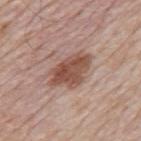{
  "biopsy_status": "not biopsied; imaged during a skin examination",
  "site": "mid back",
  "automated_metrics": {
    "area_mm2_approx": 12.0,
    "shape_asymmetry": 0.25,
    "vs_skin_darker_L": 12.0,
    "vs_skin_contrast_norm": 9.0,
    "border_irregularity_0_10": 3.0,
    "color_variation_0_10": 4.5,
    "peripheral_color_asymmetry": 1.5,
    "nevus_likeness_0_100": 85
  },
  "lesion_size": {
    "long_diameter_mm_approx": 5.5
  },
  "patient": {
    "sex": "male",
    "age_approx": 80
  },
  "image": {
    "source": "total-body photography crop",
    "field_of_view_mm": 15
  }
}